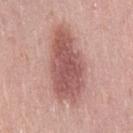Clinical impression: Part of a total-body skin-imaging series; this lesion was reviewed on a skin check and was not flagged for biopsy. Background: The recorded lesion diameter is about 9 mm. Cropped from a whole-body photographic skin survey; the tile spans about 15 mm. This is a white-light tile. The subject is a female in their 40s. The lesion is on the leg.